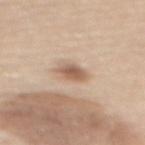Recorded during total-body skin imaging; not selected for excision or biopsy. A female subject about 65 years old. Automated image analysis of the tile measured a lesion–skin lightness drop of about 13 and a lesion-to-skin contrast of about 8 (normalized; higher = more distinct). And it measured an automated nevus-likeness rating near 85 out of 100 and a lesion-detection confidence of about 100/100. A roughly 15 mm field-of-view crop from a total-body skin photograph. The lesion's longest dimension is about 3 mm. Imaged with white-light lighting. The lesion is on the mid back.